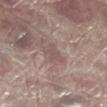{
  "biopsy_status": "not biopsied; imaged during a skin examination",
  "image": {
    "source": "total-body photography crop",
    "field_of_view_mm": 15
  },
  "site": "left lower leg",
  "patient": {
    "sex": "male",
    "age_approx": 70
  },
  "lighting": "white-light"
}